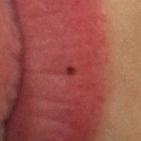The lesion was photographed on a routine skin check and not biopsied; there is no pathology result.
A region of skin cropped from a whole-body photographic capture, roughly 15 mm wide.
This is a cross-polarized tile.
An algorithmic analysis of the crop reported a shape eccentricity near 0.6 and a symmetry-axis asymmetry near 0.3. The analysis additionally found a border-irregularity rating of about 2/10, a color-variation rating of about 0/10, and peripheral color asymmetry of about 0.
Located on the head or neck.
A female patient aged approximately 25.
The recorded lesion diameter is about 1 mm.